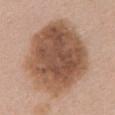The recorded lesion diameter is about 11 mm. Imaged with white-light lighting. On the mid back. A female patient aged around 65. A region of skin cropped from a whole-body photographic capture, roughly 15 mm wide. The lesion-visualizer software estimated a nevus-likeness score of about 10/100 and lesion-presence confidence of about 100/100.